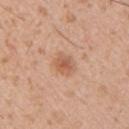No biopsy was performed on this lesion — it was imaged during a full skin examination and was not determined to be concerning. Imaged with white-light lighting. The lesion is on the left upper arm. About 3 mm across. A male patient, aged 28–32. This image is a 15 mm lesion crop taken from a total-body photograph.